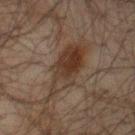Captured during whole-body skin photography for melanoma surveillance; the lesion was not biopsied.
Located on the front of the torso.
A male subject, aged 58–62.
Captured under cross-polarized illumination.
A 15 mm crop from a total-body photograph taken for skin-cancer surveillance.
Measured at roughly 8.5 mm in maximum diameter.
The total-body-photography lesion software estimated a border-irregularity index near 5/10 and radial color variation of about 2. The analysis additionally found a classifier nevus-likeness of about 80/100 and a lesion-detection confidence of about 75/100.